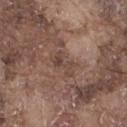{
  "biopsy_status": "not biopsied; imaged during a skin examination",
  "automated_metrics": {
    "area_mm2_approx": 5.0,
    "eccentricity": 0.85,
    "cielab_L": 44,
    "cielab_a": 17,
    "cielab_b": 23,
    "vs_skin_darker_L": 8.0,
    "vs_skin_contrast_norm": 6.0
  },
  "lighting": "white-light",
  "lesion_size": {
    "long_diameter_mm_approx": 3.5
  },
  "image": {
    "source": "total-body photography crop",
    "field_of_view_mm": 15
  },
  "site": "right thigh",
  "patient": {
    "sex": "male",
    "age_approx": 75
  }
}